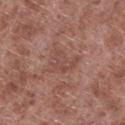Assessment: Part of a total-body skin-imaging series; this lesion was reviewed on a skin check and was not flagged for biopsy. Clinical summary: About 4 mm across. The subject is a male in their mid- to late 50s. Cropped from a total-body skin-imaging series; the visible field is about 15 mm. The lesion-visualizer software estimated a border-irregularity index near 6/10, a within-lesion color-variation index near 2.5/10, and a peripheral color-asymmetry measure near 1. From the leg.